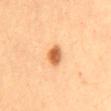The lesion was photographed on a routine skin check and not biopsied; there is no pathology result. Automated image analysis of the tile measured an eccentricity of roughly 0.8 and a shape-asymmetry score of about 0.2 (0 = symmetric). The software also gave a mean CIELAB color near L≈63 a*≈27 b*≈43, a lesion–skin lightness drop of about 16, and a lesion-to-skin contrast of about 10 (normalized; higher = more distinct). Approximately 3 mm at its widest. A female subject, aged around 65. This is a cross-polarized tile. A close-up tile cropped from a whole-body skin photograph, about 15 mm across. The lesion is located on the mid back.